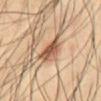The lesion was photographed on a routine skin check and not biopsied; there is no pathology result.
A male patient, about 40 years old.
About 4.5 mm across.
Located on the abdomen.
A lesion tile, about 15 mm wide, cut from a 3D total-body photograph.